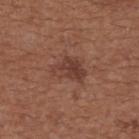The lesion was photographed on a routine skin check and not biopsied; there is no pathology result.
A lesion tile, about 15 mm wide, cut from a 3D total-body photograph.
The recorded lesion diameter is about 4 mm.
A female subject, about 65 years old.
Located on the upper back.
The total-body-photography lesion software estimated a lesion color around L≈39 a*≈21 b*≈26 in CIELAB and about 8 CIELAB-L* units darker than the surrounding skin. The analysis additionally found a within-lesion color-variation index near 4/10 and peripheral color asymmetry of about 1.5. And it measured a nevus-likeness score of about 20/100 and a lesion-detection confidence of about 100/100.
This is a white-light tile.